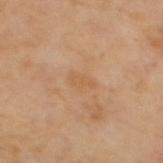notes=total-body-photography surveillance lesion; no biopsy
lesion size=~3 mm (longest diameter)
automated metrics=a mean CIELAB color near L≈60 a*≈19 b*≈38, roughly 5 lightness units darker than nearby skin, and a normalized border contrast of about 4.5; border irregularity of about 2 on a 0–10 scale and peripheral color asymmetry of about 0.5; a classifier nevus-likeness of about 0/100 and lesion-presence confidence of about 100/100
image=~15 mm tile from a whole-body skin photo
tile lighting=cross-polarized
body site=the mid back